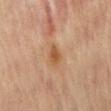Findings:
• workup: no biopsy performed (imaged during a skin exam)
• subject: male, approximately 70 years of age
• location: the lower back
• image: ~15 mm crop, total-body skin-cancer survey